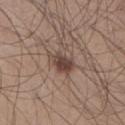The tile uses white-light illumination. A region of skin cropped from a whole-body photographic capture, roughly 15 mm wide. Located on the right lower leg. A male patient aged approximately 30.